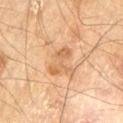lesion size = about 4 mm; subject = male, roughly 70 years of age; acquisition = ~15 mm tile from a whole-body skin photo; anatomic site = the left thigh; lighting = cross-polarized.